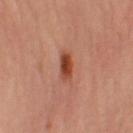{"biopsy_status": "not biopsied; imaged during a skin examination", "patient": {"sex": "female", "age_approx": 60}, "automated_metrics": {"lesion_detection_confidence_0_100": 100}, "lesion_size": {"long_diameter_mm_approx": 3.0}, "image": {"source": "total-body photography crop", "field_of_view_mm": 15}, "lighting": "cross-polarized", "site": "leg"}